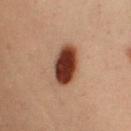Q: What is the anatomic site?
A: the arm
Q: What is the imaging modality?
A: ~15 mm tile from a whole-body skin photo
Q: Illumination type?
A: cross-polarized illumination
Q: Who is the patient?
A: female, aged 28–32
Q: Lesion size?
A: ~5 mm (longest diameter)